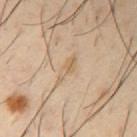biopsy_status: not biopsied; imaged during a skin examination
lesion_size:
  long_diameter_mm_approx: 4.5
patient:
  sex: male
  age_approx: 40
image:
  source: total-body photography crop
  field_of_view_mm: 15
site: right upper arm